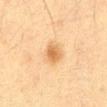Recorded during total-body skin imaging; not selected for excision or biopsy. Imaged with cross-polarized lighting. About 3 mm across. The lesion is located on the abdomen. A male patient, aged around 35. A 15 mm close-up extracted from a 3D total-body photography capture.